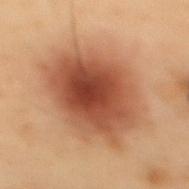Clinical impression: Captured during whole-body skin photography for melanoma surveillance; the lesion was not biopsied. Context: The lesion is located on the mid back. A male subject aged 53 to 57. Measured at roughly 9 mm in maximum diameter. A 15 mm crop from a total-body photograph taken for skin-cancer surveillance. The tile uses cross-polarized illumination.